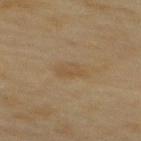| feature | finding |
|---|---|
| imaging modality | ~15 mm tile from a whole-body skin photo |
| illumination | cross-polarized |
| patient | female, roughly 60 years of age |
| lesion diameter | ≈3 mm |
| body site | the upper back |